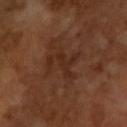Impression: The lesion was photographed on a routine skin check and not biopsied; there is no pathology result. Context: A region of skin cropped from a whole-body photographic capture, roughly 15 mm wide. Automated image analysis of the tile measured a footprint of about 7.5 mm², an outline eccentricity of about 0.8 (0 = round, 1 = elongated), and two-axis asymmetry of about 0.6. The software also gave a lesion color around L≈27 a*≈19 b*≈26 in CIELAB, a lesion–skin lightness drop of about 5, and a normalized border contrast of about 6. The software also gave radial color variation of about 0. A male subject roughly 65 years of age.